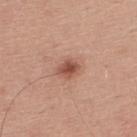Q: Was a biopsy performed?
A: catalogued during a skin exam; not biopsied
Q: Automated lesion metrics?
A: an area of roughly 4 mm², a shape eccentricity near 0.7, and a symmetry-axis asymmetry near 0.25; a normalized lesion–skin contrast near 8.5; a nevus-likeness score of about 90/100
Q: What is the imaging modality?
A: ~15 mm tile from a whole-body skin photo
Q: Patient demographics?
A: male, aged approximately 30
Q: What is the anatomic site?
A: the upper back